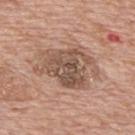biopsy_status: not biopsied; imaged during a skin examination
image:
  source: total-body photography crop
  field_of_view_mm: 15
site: upper back
patient:
  sex: male
  age_approx: 70
automated_metrics:
  eccentricity: 0.75
  shape_asymmetry: 0.35
  cielab_L: 53
  cielab_a: 18
  cielab_b: 27
  vs_skin_contrast_norm: 7.5
  border_irregularity_0_10: 5.5
  color_variation_0_10: 6.0
  peripheral_color_asymmetry: 2.5
  nevus_likeness_0_100: 0
  lesion_detection_confidence_0_100: 100